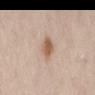<lesion>
  <biopsy_status>not biopsied; imaged during a skin examination</biopsy_status>
  <patient>
    <sex>male</sex>
    <age_approx>80</age_approx>
  </patient>
  <lesion_size>
    <long_diameter_mm_approx>3.0</long_diameter_mm_approx>
  </lesion_size>
  <site>mid back</site>
  <image>
    <source>total-body photography crop</source>
    <field_of_view_mm>15</field_of_view_mm>
  </image>
  <lighting>white-light</lighting>
</lesion>